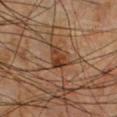Notes:
– follow-up: catalogued during a skin exam; not biopsied
– site: the chest
– patient: male, aged 48 to 52
– acquisition: ~15 mm crop, total-body skin-cancer survey
– lighting: cross-polarized